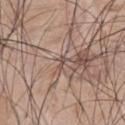| key | value |
|---|---|
| workup | no biopsy performed (imaged during a skin exam) |
| size | about 1.5 mm |
| image source | total-body-photography crop, ~15 mm field of view |
| site | the chest |
| automated metrics | a shape eccentricity near 0.9 and a shape-asymmetry score of about 0.45 (0 = symmetric) |
| subject | male, aged 43 to 47 |
| tile lighting | white-light illumination |